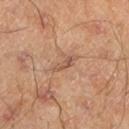{"biopsy_status": "not biopsied; imaged during a skin examination", "lighting": "cross-polarized", "patient": {"sex": "male", "age_approx": 45}, "site": "left lower leg", "lesion_size": {"long_diameter_mm_approx": 2.5}, "image": {"source": "total-body photography crop", "field_of_view_mm": 15}}